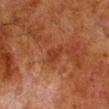Notes:
– workup: total-body-photography surveillance lesion; no biopsy
– image: total-body-photography crop, ~15 mm field of view
– subject: male, roughly 80 years of age
– site: the right lower leg